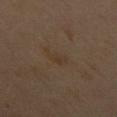workup: catalogued during a skin exam; not biopsied
subject: male, aged 58–62
imaging modality: ~15 mm tile from a whole-body skin photo
site: the mid back